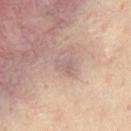Q: Was this lesion biopsied?
A: catalogued during a skin exam; not biopsied
Q: Lesion location?
A: the upper back
Q: What are the patient's age and sex?
A: male, in their mid- to late 30s
Q: What is the imaging modality?
A: 15 mm crop, total-body photography
Q: Lesion size?
A: about 2.5 mm
Q: How was the tile lit?
A: cross-polarized